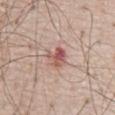Assessment: The lesion was photographed on a routine skin check and not biopsied; there is no pathology result.